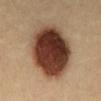A 15 mm crop from a total-body photograph taken for skin-cancer surveillance. A female subject, aged 38 to 42. Imaged with cross-polarized lighting. On the abdomen.